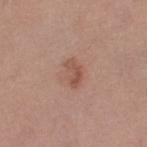No biopsy was performed on this lesion — it was imaged during a full skin examination and was not determined to be concerning.